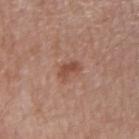<tbp_lesion>
<image>
  <source>total-body photography crop</source>
  <field_of_view_mm>15</field_of_view_mm>
</image>
<site>left forearm</site>
<lighting>white-light</lighting>
<patient>
  <sex>female</sex>
  <age_approx>50</age_approx>
</patient>
<lesion_size>
  <long_diameter_mm_approx>2.5</long_diameter_mm_approx>
</lesion_size>
<automated_metrics>
  <area_mm2_approx>4.0</area_mm2_approx>
  <eccentricity>0.75</eccentricity>
  <shape_asymmetry>0.3</shape_asymmetry>
  <vs_skin_darker_L>9.0</vs_skin_darker_L>
  <vs_skin_contrast_norm>6.5</vs_skin_contrast_norm>
  <border_irregularity_0_10>3.5</border_irregularity_0_10>
  <color_variation_0_10>2.0</color_variation_0_10>
  <peripheral_color_asymmetry>0.5</peripheral_color_asymmetry>
</automated_metrics>
</tbp_lesion>